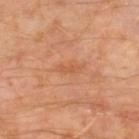Notes:
– follow-up · catalogued during a skin exam; not biopsied
– patient · male, aged around 60
– imaging modality · 15 mm crop, total-body photography
– diameter · about 3 mm
– illumination · cross-polarized illumination
– anatomic site · the right lower leg